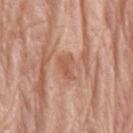<lesion>
  <biopsy_status>not biopsied; imaged during a skin examination</biopsy_status>
  <site>arm</site>
  <patient>
    <sex>female</sex>
    <age_approx>75</age_approx>
  </patient>
  <lesion_size>
    <long_diameter_mm_approx>2.5</long_diameter_mm_approx>
  </lesion_size>
  <automated_metrics>
    <area_mm2_approx>4.0</area_mm2_approx>
    <eccentricity>0.75</eccentricity>
    <shape_asymmetry>0.5</shape_asymmetry>
    <cielab_L>56</cielab_L>
    <cielab_a>23</cielab_a>
    <cielab_b>31</cielab_b>
    <vs_skin_contrast_norm>5.5</vs_skin_contrast_norm>
  </automated_metrics>
  <image>
    <source>total-body photography crop</source>
    <field_of_view_mm>15</field_of_view_mm>
  </image>
  <lighting>white-light</lighting>
</lesion>